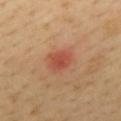Imaged during a routine full-body skin examination; the lesion was not biopsied and no histopathology is available. Located on the upper back. Captured under cross-polarized illumination. Automated image analysis of the tile measured a lesion color around L≈49 a*≈29 b*≈32 in CIELAB and about 9 CIELAB-L* units darker than the surrounding skin. Measured at roughly 3 mm in maximum diameter. The patient is a female aged 48 to 52. A 15 mm crop from a total-body photograph taken for skin-cancer surveillance.